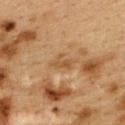Clinical impression:
The lesion was tiled from a total-body skin photograph and was not biopsied.
Background:
The lesion-visualizer software estimated a border-irregularity index near 5.5/10, a color-variation rating of about 2.5/10, and peripheral color asymmetry of about 0.5. The lesion is on the upper back. Imaged with cross-polarized lighting. A region of skin cropped from a whole-body photographic capture, roughly 15 mm wide. The subject is a female aged 38–42. Approximately 3 mm at its widest.